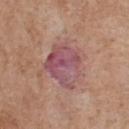Clinical impression: Recorded during total-body skin imaging; not selected for excision or biopsy. Background: From the upper back. The subject is a male aged approximately 60. The lesion's longest dimension is about 6 mm. The tile uses white-light illumination. The total-body-photography lesion software estimated a shape eccentricity near 0.35 and a shape-asymmetry score of about 0.3 (0 = symmetric). And it measured a mean CIELAB color near L≈52 a*≈24 b*≈19, roughly 8 lightness units darker than nearby skin, and a lesion-to-skin contrast of about 8.5 (normalized; higher = more distinct). The software also gave a border-irregularity rating of about 4.5/10, internal color variation of about 8 on a 0–10 scale, and radial color variation of about 2.5. A 15 mm close-up tile from a total-body photography series done for melanoma screening.